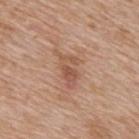Recorded during total-body skin imaging; not selected for excision or biopsy.